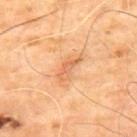biopsy status: total-body-photography surveillance lesion; no biopsy
tile lighting: cross-polarized
location: the upper back
patient: male, in their mid- to late 60s
image: ~15 mm tile from a whole-body skin photo
diameter: ~3.5 mm (longest diameter)